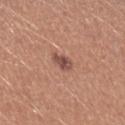This lesion was catalogued during total-body skin photography and was not selected for biopsy.
Cropped from a total-body skin-imaging series; the visible field is about 15 mm.
The tile uses white-light illumination.
Measured at roughly 2.5 mm in maximum diameter.
A female patient in their mid-20s.
An algorithmic analysis of the crop reported a footprint of about 3.5 mm², a shape eccentricity near 0.7, and two-axis asymmetry of about 0.3. The analysis additionally found a nevus-likeness score of about 70/100 and a detector confidence of about 100 out of 100 that the crop contains a lesion.
On the left upper arm.